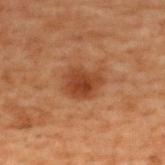Captured during whole-body skin photography for melanoma surveillance; the lesion was not biopsied. Imaged with cross-polarized lighting. This image is a 15 mm lesion crop taken from a total-body photograph. The total-body-photography lesion software estimated a mean CIELAB color near L≈33 a*≈21 b*≈29, a lesion–skin lightness drop of about 8, and a normalized lesion–skin contrast near 8. And it measured a nevus-likeness score of about 85/100 and a detector confidence of about 100 out of 100 that the crop contains a lesion. A male patient, aged approximately 70. On the back. The lesion's longest dimension is about 4 mm.